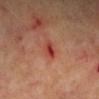Assessment:
The lesion was tiled from a total-body skin photograph and was not biopsied.
Context:
The subject is a male about 65 years old. Captured under cross-polarized illumination. The lesion's longest dimension is about 2.5 mm. From the right lower leg. A 15 mm close-up extracted from a 3D total-body photography capture.